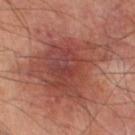notes=no biopsy performed (imaged during a skin exam) | lighting=cross-polarized illumination | image source=~15 mm tile from a whole-body skin photo | lesion size=~9 mm (longest diameter) | automated lesion analysis=a border-irregularity index near 5/10, internal color variation of about 6 on a 0–10 scale, and radial color variation of about 1.5 | subject=male, aged 63 to 67 | anatomic site=the left lower leg.